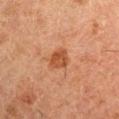Q: Was a biopsy performed?
A: catalogued during a skin exam; not biopsied
Q: What is the anatomic site?
A: the arm
Q: What lighting was used for the tile?
A: cross-polarized
Q: How large is the lesion?
A: ≈2.5 mm
Q: What did automated image analysis measure?
A: an average lesion color of about L≈39 a*≈22 b*≈31 (CIELAB), roughly 8 lightness units darker than nearby skin, and a normalized border contrast of about 7.5
Q: Who is the patient?
A: male, aged approximately 50
Q: What is the imaging modality?
A: ~15 mm tile from a whole-body skin photo